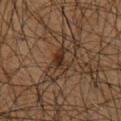This lesion was catalogued during total-body skin photography and was not selected for biopsy.
A male subject, aged around 65.
Approximately 3 mm at its widest.
From the chest.
The tile uses cross-polarized illumination.
Automated image analysis of the tile measured a border-irregularity index near 5/10, a color-variation rating of about 2.5/10, and a peripheral color-asymmetry measure near 0.5. The software also gave a nevus-likeness score of about 50/100 and a lesion-detection confidence of about 85/100.
A region of skin cropped from a whole-body photographic capture, roughly 15 mm wide.